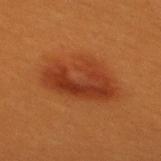follow-up: catalogued during a skin exam; not biopsied | anatomic site: the back | TBP lesion metrics: a footprint of about 24 mm² and an eccentricity of roughly 0.8; about 9 CIELAB-L* units darker than the surrounding skin; a border-irregularity index near 2.5/10, a color-variation rating of about 6/10, and peripheral color asymmetry of about 2 | patient: female, in their 30s | lesion size: ≈7.5 mm | image source: total-body-photography crop, ~15 mm field of view.